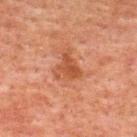Findings:
– notes: imaged on a skin check; not biopsied
– anatomic site: the back
– diameter: ~3 mm (longest diameter)
– image source: ~15 mm crop, total-body skin-cancer survey
– lighting: cross-polarized
– patient: male, aged 58 to 62